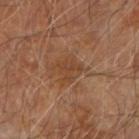Imaged during a routine full-body skin examination; the lesion was not biopsied and no histopathology is available. The lesion-visualizer software estimated a lesion area of about 4.5 mm² and a symmetry-axis asymmetry near 0.55. It also reported a lesion color around L≈41 a*≈20 b*≈32 in CIELAB, a lesion–skin lightness drop of about 6, and a normalized lesion–skin contrast near 5. The analysis additionally found a border-irregularity rating of about 6.5/10. And it measured a nevus-likeness score of about 0/100 and lesion-presence confidence of about 100/100. This is a cross-polarized tile. Cropped from a whole-body photographic skin survey; the tile spans about 15 mm. Located on the arm. A male patient, about 70 years old.